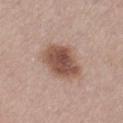Case summary:
- biopsy status · imaged on a skin check; not biopsied
- subject · male, in their mid-60s
- location · the left thigh
- imaging modality · total-body-photography crop, ~15 mm field of view
- lesion size · about 4.5 mm
- lighting · white-light illumination